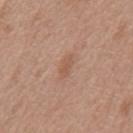<tbp_lesion>
  <site>mid back</site>
  <image>
    <source>total-body photography crop</source>
    <field_of_view_mm>15</field_of_view_mm>
  </image>
  <lesion_size>
    <long_diameter_mm_approx>3.0</long_diameter_mm_approx>
  </lesion_size>
  <patient>
    <sex>female</sex>
    <age_approx>75</age_approx>
  </patient>
  <lighting>white-light</lighting>
  <automated_metrics>
    <area_mm2_approx>3.0</area_mm2_approx>
    <eccentricity>0.9</eccentricity>
    <shape_asymmetry>0.3</shape_asymmetry>
    <cielab_L>55</cielab_L>
    <cielab_a>20</cielab_a>
    <cielab_b>30</cielab_b>
    <vs_skin_darker_L>7.0</vs_skin_darker_L>
    <vs_skin_contrast_norm>5.5</vs_skin_contrast_norm>
    <border_irregularity_0_10>3.0</border_irregularity_0_10>
    <color_variation_0_10>2.5</color_variation_0_10>
    <peripheral_color_asymmetry>1.5</peripheral_color_asymmetry>
  </automated_metrics>
</tbp_lesion>